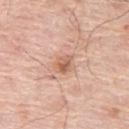Q: Was a biopsy performed?
A: catalogued during a skin exam; not biopsied
Q: How was the tile lit?
A: white-light illumination
Q: Patient demographics?
A: male, aged approximately 80
Q: What did automated image analysis measure?
A: a nevus-likeness score of about 35/100 and lesion-presence confidence of about 100/100
Q: How was this image acquired?
A: ~15 mm tile from a whole-body skin photo
Q: Lesion location?
A: the left thigh